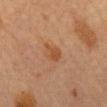The lesion was tiled from a total-body skin photograph and was not biopsied. Imaged with cross-polarized lighting. The lesion's longest dimension is about 3 mm. A female patient approximately 60 years of age. The lesion is on the mid back. Cropped from a total-body skin-imaging series; the visible field is about 15 mm.